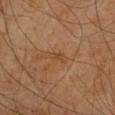| feature | finding |
|---|---|
| patient | male, aged around 70 |
| body site | the right forearm |
| tile lighting | cross-polarized illumination |
| acquisition | ~15 mm tile from a whole-body skin photo |